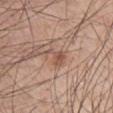notes: total-body-photography surveillance lesion; no biopsy
image: total-body-photography crop, ~15 mm field of view
patient: male, roughly 50 years of age
size: about 3 mm
anatomic site: the arm
illumination: white-light illumination